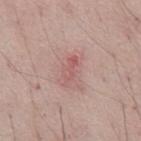Assessment: This lesion was catalogued during total-body skin photography and was not selected for biopsy. Acquisition and patient details: The lesion's longest dimension is about 3.5 mm. Captured under white-light illumination. A lesion tile, about 15 mm wide, cut from a 3D total-body photograph. The patient is a male approximately 65 years of age. The lesion is on the abdomen.